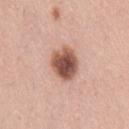The lesion-visualizer software estimated a lesion area of about 10 mm², a shape eccentricity near 0.35, and a symmetry-axis asymmetry near 0.15.
The lesion is on the lower back.
This image is a 15 mm lesion crop taken from a total-body photograph.
Imaged with white-light lighting.
A female subject aged 28 to 32.
Longest diameter approximately 3.5 mm.